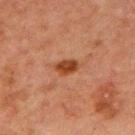{"biopsy_status": "not biopsied; imaged during a skin examination"}